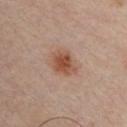Assessment: Part of a total-body skin-imaging series; this lesion was reviewed on a skin check and was not flagged for biopsy. Background: The lesion is on the chest. A male subject in their mid- to late 30s. A close-up tile cropped from a whole-body skin photograph, about 15 mm across. The lesion-visualizer software estimated a footprint of about 8.5 mm², an eccentricity of roughly 0.55, and two-axis asymmetry of about 0.2. Measured at roughly 3.5 mm in maximum diameter.